The patient is a female aged 28–32. A close-up tile cropped from a whole-body skin photograph, about 15 mm across. The lesion's longest dimension is about 2.5 mm. The lesion is on the leg. This is a cross-polarized tile: 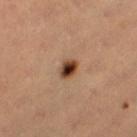The lesion was biopsied, and histopathology showed a junctional melanocytic nevus, classified as a benign skin lesion.